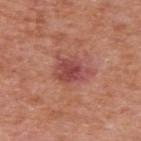Q: Was this lesion biopsied?
A: catalogued during a skin exam; not biopsied
Q: Where on the body is the lesion?
A: the upper back
Q: Patient demographics?
A: male, about 65 years old
Q: What kind of image is this?
A: 15 mm crop, total-body photography
Q: How was the tile lit?
A: white-light
Q: Automated lesion metrics?
A: an outline eccentricity of about 0.75 (0 = round, 1 = elongated); a lesion–skin lightness drop of about 10 and a lesion-to-skin contrast of about 7.5 (normalized; higher = more distinct); a border-irregularity index near 3.5/10, a within-lesion color-variation index near 4.5/10, and radial color variation of about 1.5; an automated nevus-likeness rating near 5 out of 100 and lesion-presence confidence of about 100/100
Q: Lesion size?
A: ≈4.5 mm